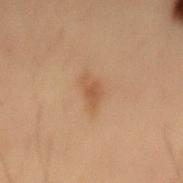Image and clinical context:
This is a cross-polarized tile. This image is a 15 mm lesion crop taken from a total-body photograph. On the lower back. Automated tile analysis of the lesion measured a mean CIELAB color near L≈43 a*≈17 b*≈29, about 7 CIELAB-L* units darker than the surrounding skin, and a normalized border contrast of about 6. The software also gave an automated nevus-likeness rating near 25 out of 100 and lesion-presence confidence of about 100/100. The subject is a male roughly 55 years of age.